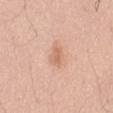A 15 mm crop from a total-body photograph taken for skin-cancer surveillance.
Measured at roughly 2.5 mm in maximum diameter.
The lesion is located on the abdomen.
A male subject aged 48 to 52.
Captured under white-light illumination.